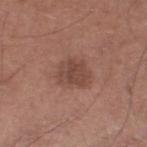biopsy_status: not biopsied; imaged during a skin examination
site: right lower leg
patient:
  sex: male
  age_approx: 65
image:
  source: total-body photography crop
  field_of_view_mm: 15